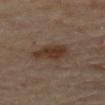The lesion was photographed on a routine skin check and not biopsied; there is no pathology result.
A female patient, aged approximately 60.
On the upper back.
A 15 mm close-up extracted from a 3D total-body photography capture.
Captured under cross-polarized illumination.
Approximately 5 mm at its widest.